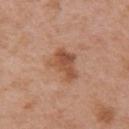biopsy status: no biopsy performed (imaged during a skin exam)
imaging modality: 15 mm crop, total-body photography
TBP lesion metrics: a symmetry-axis asymmetry near 0.35; an average lesion color of about L≈52 a*≈23 b*≈33 (CIELAB); a border-irregularity index near 3.5/10, internal color variation of about 4.5 on a 0–10 scale, and a peripheral color-asymmetry measure near 1.5; a classifier nevus-likeness of about 20/100 and a lesion-detection confidence of about 100/100
patient: female, aged 48–52
body site: the left upper arm